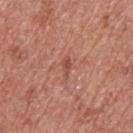Q: What kind of image is this?
A: ~15 mm crop, total-body skin-cancer survey
Q: What are the patient's age and sex?
A: male, aged 68 to 72
Q: Lesion location?
A: the upper back